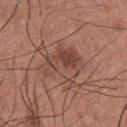Part of a total-body skin-imaging series; this lesion was reviewed on a skin check and was not flagged for biopsy. Approximately 5.5 mm at its widest. A region of skin cropped from a whole-body photographic capture, roughly 15 mm wide. The subject is a male aged 33–37. Automated tile analysis of the lesion measured a footprint of about 15 mm², a shape eccentricity near 0.55, and a symmetry-axis asymmetry near 0.4. The software also gave about 8 CIELAB-L* units darker than the surrounding skin and a normalized border contrast of about 6. The software also gave a nevus-likeness score of about 75/100 and lesion-presence confidence of about 100/100. The lesion is on the chest.